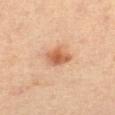| field | value |
|---|---|
| follow-up | total-body-photography surveillance lesion; no biopsy |
| lesion diameter | about 3 mm |
| tile lighting | cross-polarized |
| patient | female, approximately 50 years of age |
| automated lesion analysis | a footprint of about 6 mm² and a shape eccentricity near 0.6; a mean CIELAB color near L≈59 a*≈24 b*≈36, a lesion–skin lightness drop of about 12, and a lesion-to-skin contrast of about 8 (normalized; higher = more distinct) |
| body site | the right thigh |
| image | 15 mm crop, total-body photography |